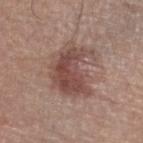The lesion was photographed on a routine skin check and not biopsied; there is no pathology result. Measured at roughly 6 mm in maximum diameter. The lesion is on the right lower leg. A male patient, aged approximately 65. This image is a 15 mm lesion crop taken from a total-body photograph. This is a white-light tile.